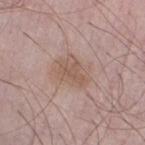Q: Is there a histopathology result?
A: imaged on a skin check; not biopsied
Q: Illumination type?
A: white-light illumination
Q: What did automated image analysis measure?
A: border irregularity of about 3.5 on a 0–10 scale, a color-variation rating of about 2.5/10, and radial color variation of about 1
Q: What is the imaging modality?
A: 15 mm crop, total-body photography
Q: Lesion location?
A: the left thigh
Q: Who is the patient?
A: male, in their mid- to late 60s
Q: Lesion size?
A: ≈4 mm